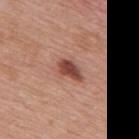follow-up = imaged on a skin check; not biopsied | patient = female, aged 58–62 | anatomic site = the back | acquisition = ~15 mm crop, total-body skin-cancer survey | lighting = white-light illumination.